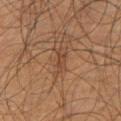Background: Cropped from a total-body skin-imaging series; the visible field is about 15 mm. The patient is a male about 60 years old. The lesion's longest dimension is about 3 mm. Located on the back. Automated image analysis of the tile measured a lesion area of about 3.5 mm². And it measured a mean CIELAB color near L≈39 a*≈18 b*≈28, about 6 CIELAB-L* units darker than the surrounding skin, and a lesion-to-skin contrast of about 5.5 (normalized; higher = more distinct). It also reported a border-irregularity index near 4.5/10, internal color variation of about 2 on a 0–10 scale, and radial color variation of about 0.5.